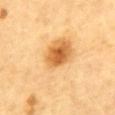Assessment: The lesion was photographed on a routine skin check and not biopsied; there is no pathology result. Clinical summary: A male subject aged 83 or over. Approximately 9 mm at its widest. A roughly 15 mm field-of-view crop from a total-body skin photograph. From the front of the torso.